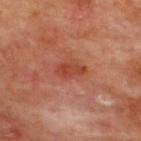Q: Was a biopsy performed?
A: imaged on a skin check; not biopsied
Q: What is the lesion's diameter?
A: ≈3.5 mm
Q: What are the patient's age and sex?
A: male, approximately 65 years of age
Q: Where on the body is the lesion?
A: the upper back
Q: What lighting was used for the tile?
A: cross-polarized illumination
Q: What is the imaging modality?
A: total-body-photography crop, ~15 mm field of view